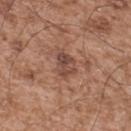{
  "biopsy_status": "not biopsied; imaged during a skin examination",
  "lesion_size": {
    "long_diameter_mm_approx": 3.0
  },
  "site": "upper back",
  "image": {
    "source": "total-body photography crop",
    "field_of_view_mm": 15
  },
  "lighting": "white-light",
  "patient": {
    "sex": "male",
    "age_approx": 55
  }
}